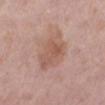Image and clinical context:
The lesion is on the left lower leg. A female patient aged around 40. A 15 mm close-up extracted from a 3D total-body photography capture. About 5.5 mm across. The total-body-photography lesion software estimated a lesion area of about 16 mm², a shape eccentricity near 0.7, and a symmetry-axis asymmetry near 0.2. And it measured a border-irregularity rating of about 2.5/10, internal color variation of about 4 on a 0–10 scale, and a peripheral color-asymmetry measure near 1.5. It also reported an automated nevus-likeness rating near 0 out of 100 and lesion-presence confidence of about 100/100. Imaged with white-light lighting.